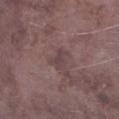The lesion was tiled from a total-body skin photograph and was not biopsied. The lesion is on the left lower leg. The subject is a male approximately 75 years of age. A roughly 15 mm field-of-view crop from a total-body skin photograph.